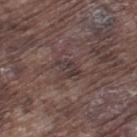patient=male, aged approximately 75
lesion diameter=~2.5 mm (longest diameter)
automated lesion analysis=an average lesion color of about L≈35 a*≈14 b*≈15 (CIELAB), a lesion–skin lightness drop of about 7, and a normalized border contrast of about 7; a border-irregularity index near 5.5/10, internal color variation of about 0 on a 0–10 scale, and radial color variation of about 0
illumination=white-light
anatomic site=the left thigh
image=~15 mm tile from a whole-body skin photo